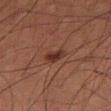Case summary:
* biopsy status: imaged on a skin check; not biopsied
* lesion diameter: ~3 mm (longest diameter)
* image source: ~15 mm crop, total-body skin-cancer survey
* patient: male, in their mid-50s
* body site: the left thigh